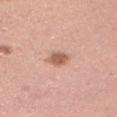Image and clinical context:
The total-body-photography lesion software estimated a footprint of about 4.5 mm², a shape eccentricity near 0.6, and a shape-asymmetry score of about 0.2 (0 = symmetric). It also reported a border-irregularity rating of about 1.5/10 and a within-lesion color-variation index near 2.5/10. The software also gave an automated nevus-likeness rating near 85 out of 100 and a detector confidence of about 100 out of 100 that the crop contains a lesion. From the left upper arm. A male subject approximately 35 years of age. A 15 mm close-up extracted from a 3D total-body photography capture.